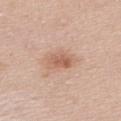Notes:
- notes — no biopsy performed (imaged during a skin exam)
- acquisition — ~15 mm crop, total-body skin-cancer survey
- lesion size — about 3.5 mm
- location — the upper back
- tile lighting — white-light illumination
- patient — female, aged 48–52